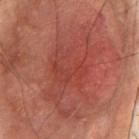The lesion was tiled from a total-body skin photograph and was not biopsied.
About 9.5 mm across.
The patient is a male aged 53 to 57.
The tile uses cross-polarized illumination.
On the chest.
A 15 mm close-up extracted from a 3D total-body photography capture.
Automated image analysis of the tile measured an area of roughly 32 mm², a shape eccentricity near 0.85, and a symmetry-axis asymmetry near 0.4. The software also gave an average lesion color of about L≈45 a*≈31 b*≈29 (CIELAB) and roughly 7 lightness units darker than nearby skin. The software also gave a color-variation rating of about 4.5/10 and peripheral color asymmetry of about 1.5.